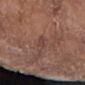Imaged during a routine full-body skin examination; the lesion was not biopsied and no histopathology is available.
Measured at roughly 3 mm in maximum diameter.
Cropped from a whole-body photographic skin survey; the tile spans about 15 mm.
A female patient, aged 73–77.
From the left forearm.
Captured under white-light illumination.